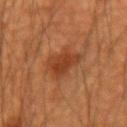Findings:
* notes · imaged on a skin check; not biopsied
* patient · male, in their mid-50s
* location · the left upper arm
* diameter · ~4 mm (longest diameter)
* imaging modality · total-body-photography crop, ~15 mm field of view
* lighting · cross-polarized illumination